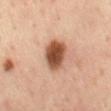Notes:
• workup: no biopsy performed (imaged during a skin exam)
• image source: ~15 mm crop, total-body skin-cancer survey
• subject: male, in their mid- to late 50s
• site: the mid back
• automated metrics: a footprint of about 11 mm², a shape eccentricity near 0.65, and two-axis asymmetry of about 0.15; a color-variation rating of about 5.5/10 and peripheral color asymmetry of about 1.5; a nevus-likeness score of about 100/100
• tile lighting: cross-polarized
• diameter: about 4 mm